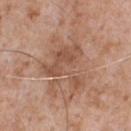notes: catalogued during a skin exam; not biopsied | size: ~6 mm (longest diameter) | lighting: white-light illumination | image-analysis metrics: a footprint of about 16 mm², a shape eccentricity near 0.5, and a shape-asymmetry score of about 0.6 (0 = symmetric); border irregularity of about 8 on a 0–10 scale and peripheral color asymmetry of about 1; a nevus-likeness score of about 0/100 and a detector confidence of about 100 out of 100 that the crop contains a lesion | location: the chest | image source: 15 mm crop, total-body photography | subject: male, approximately 65 years of age.